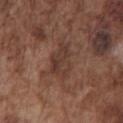| field | value |
|---|---|
| TBP lesion metrics | a lesion-detection confidence of about 65/100 |
| anatomic site | the chest |
| image source | 15 mm crop, total-body photography |
| patient | male, roughly 75 years of age |